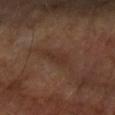follow-up: total-body-photography surveillance lesion; no biopsy | tile lighting: cross-polarized illumination | site: the left forearm | acquisition: ~15 mm crop, total-body skin-cancer survey | TBP lesion metrics: an area of roughly 4.5 mm², a shape eccentricity near 0.85, and two-axis asymmetry of about 0.35; a lesion–skin lightness drop of about 5 and a lesion-to-skin contrast of about 5.5 (normalized; higher = more distinct); a border-irregularity index near 3.5/10, a color-variation rating of about 1.5/10, and peripheral color asymmetry of about 0.5 | lesion size: ~3.5 mm (longest diameter) | patient: female, aged 58–62.